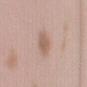lesion size: about 3 mm
body site: the chest
patient: female, aged 23 to 27
imaging modality: 15 mm crop, total-body photography
illumination: white-light
TBP lesion metrics: a shape eccentricity near 0.8; a nevus-likeness score of about 80/100